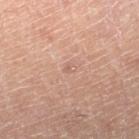Clinical impression:
Part of a total-body skin-imaging series; this lesion was reviewed on a skin check and was not flagged for biopsy.
Acquisition and patient details:
A male patient, in their mid-50s. The lesion's longest dimension is about 1.5 mm. From the right lower leg. This image is a 15 mm lesion crop taken from a total-body photograph.